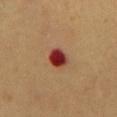diameter: ≈3 mm | anatomic site: the chest | illumination: cross-polarized illumination | patient: male, aged 58–62 | acquisition: ~15 mm crop, total-body skin-cancer survey.